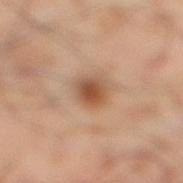- notes: imaged on a skin check; not biopsied
- illumination: cross-polarized
- image source: ~15 mm tile from a whole-body skin photo
- anatomic site: the right lower leg
- image-analysis metrics: an automated nevus-likeness rating near 90 out of 100
- lesion diameter: ≈2.5 mm
- subject: male, aged approximately 55